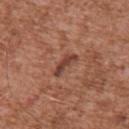Assessment:
Recorded during total-body skin imaging; not selected for excision or biopsy.
Background:
A 15 mm crop from a total-body photograph taken for skin-cancer surveillance. The lesion is located on the chest. A male subject aged around 45.